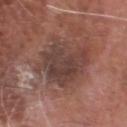Clinical impression: Captured during whole-body skin photography for melanoma surveillance; the lesion was not biopsied. Context: The lesion's longest dimension is about 7.5 mm. The lesion is on the head or neck. The lesion-visualizer software estimated a lesion area of about 23 mm² and a shape-asymmetry score of about 0.2 (0 = symmetric). It also reported roughly 9 lightness units darker than nearby skin. The analysis additionally found border irregularity of about 4 on a 0–10 scale and a color-variation rating of about 4/10. The analysis additionally found an automated nevus-likeness rating near 0 out of 100 and lesion-presence confidence of about 100/100. A 15 mm close-up tile from a total-body photography series done for melanoma screening. A male subject in their mid- to late 70s.